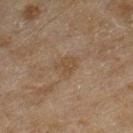follow-up: total-body-photography surveillance lesion; no biopsy
anatomic site: the right lower leg
patient: female, aged 58 to 62
acquisition: ~15 mm tile from a whole-body skin photo
illumination: cross-polarized illumination
size: ≈3 mm